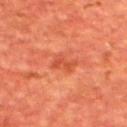- follow-up · catalogued during a skin exam; not biopsied
- lesion diameter · ≈3 mm
- location · the back
- illumination · cross-polarized illumination
- subject · male, aged 63–67
- image · ~15 mm tile from a whole-body skin photo
- automated metrics · a footprint of about 4.5 mm², a shape eccentricity near 0.7, and two-axis asymmetry of about 0.4; a mean CIELAB color near L≈48 a*≈34 b*≈38, a lesion–skin lightness drop of about 7, and a normalized border contrast of about 5.5; a nevus-likeness score of about 0/100